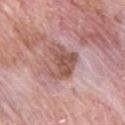{"biopsy_status": "not biopsied; imaged during a skin examination", "site": "back", "patient": {"sex": "male", "age_approx": 60}, "image": {"source": "total-body photography crop", "field_of_view_mm": 15}}